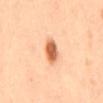Recorded during total-body skin imaging; not selected for excision or biopsy. Located on the back. A female subject aged around 35. A close-up tile cropped from a whole-body skin photograph, about 15 mm across. The total-body-photography lesion software estimated a lesion color around L≈67 a*≈27 b*≈41 in CIELAB, about 17 CIELAB-L* units darker than the surrounding skin, and a normalized lesion–skin contrast near 9.5. It also reported a border-irregularity index near 2.5/10, a color-variation rating of about 5.5/10, and a peripheral color-asymmetry measure near 1.5. The tile uses cross-polarized illumination.